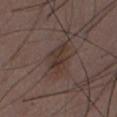The lesion was photographed on a routine skin check and not biopsied; there is no pathology result. Imaged with white-light lighting. The lesion's longest dimension is about 4.5 mm. A male subject in their 50s. The lesion-visualizer software estimated an area of roughly 6 mm², an eccentricity of roughly 0.9, and a shape-asymmetry score of about 0.4 (0 = symmetric). It also reported a lesion–skin lightness drop of about 7 and a normalized lesion–skin contrast near 7.5. It also reported a classifier nevus-likeness of about 15/100. From the abdomen. A 15 mm crop from a total-body photograph taken for skin-cancer surveillance.